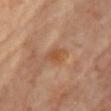biopsy_status: not biopsied; imaged during a skin examination
image:
  source: total-body photography crop
  field_of_view_mm: 15
site: chest
lesion_size:
  long_diameter_mm_approx: 3.0
lighting: cross-polarized
patient:
  sex: female
  age_approx: 60